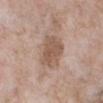Captured during whole-body skin photography for melanoma surveillance; the lesion was not biopsied.
A close-up tile cropped from a whole-body skin photograph, about 15 mm across.
The tile uses white-light illumination.
On the right lower leg.
Measured at roughly 4 mm in maximum diameter.
The subject is a female roughly 55 years of age.